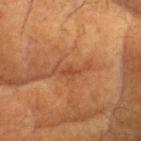Captured during whole-body skin photography for melanoma surveillance; the lesion was not biopsied. A female patient aged around 80. A region of skin cropped from a whole-body photographic capture, roughly 15 mm wide. The lesion is located on the head or neck. Automated tile analysis of the lesion measured a normalized border contrast of about 5. The analysis additionally found a border-irregularity index near 6.5/10, a within-lesion color-variation index near 1.5/10, and peripheral color asymmetry of about 0. And it measured a classifier nevus-likeness of about 0/100. This is a cross-polarized tile. The lesion's longest dimension is about 4.5 mm.